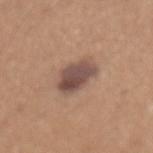The patient is a female about 35 years old. A lesion tile, about 15 mm wide, cut from a 3D total-body photograph. Approximately 4 mm at its widest. Imaged with white-light lighting. The lesion is on the mid back.